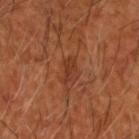Q: Is there a histopathology result?
A: imaged on a skin check; not biopsied
Q: What is the imaging modality?
A: total-body-photography crop, ~15 mm field of view
Q: Illumination type?
A: cross-polarized illumination
Q: What are the patient's age and sex?
A: male, in their mid-60s